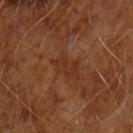No biopsy was performed on this lesion — it was imaged during a full skin examination and was not determined to be concerning. The subject is a male in their mid- to late 60s. A 15 mm close-up tile from a total-body photography series done for melanoma screening.